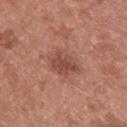Captured during whole-body skin photography for melanoma surveillance; the lesion was not biopsied. The lesion is on the upper back. This is a white-light tile. A close-up tile cropped from a whole-body skin photograph, about 15 mm across. A female patient, approximately 40 years of age. About 4 mm across.